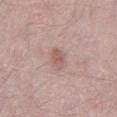Clinical impression: Imaged during a routine full-body skin examination; the lesion was not biopsied and no histopathology is available. Acquisition and patient details: The tile uses white-light illumination. A 15 mm close-up extracted from a 3D total-body photography capture. About 3.5 mm across. The patient is a male in their 30s.